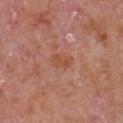Impression: Part of a total-body skin-imaging series; this lesion was reviewed on a skin check and was not flagged for biopsy. Clinical summary: The lesion is located on the chest. The recorded lesion diameter is about 3 mm. The patient is a male aged 63–67. The tile uses white-light illumination. A region of skin cropped from a whole-body photographic capture, roughly 15 mm wide.